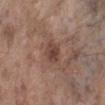lighting: white-light
patient: female, aged around 85 or older
body site: the left lower leg
TBP lesion metrics: a border-irregularity index near 1.5/10, a within-lesion color-variation index near 2.5/10, and radial color variation of about 1; a classifier nevus-likeness of about 35/100 and lesion-presence confidence of about 100/100
image source: 15 mm crop, total-body photography
lesion size: ~2.5 mm (longest diameter)